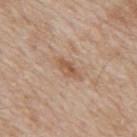<tbp_lesion>
<biopsy_status>not biopsied; imaged during a skin examination</biopsy_status>
<image>
  <source>total-body photography crop</source>
  <field_of_view_mm>15</field_of_view_mm>
</image>
<patient>
  <sex>male</sex>
  <age_approx>65</age_approx>
</patient>
<lighting>white-light</lighting>
<lesion_size>
  <long_diameter_mm_approx>3.0</long_diameter_mm_approx>
</lesion_size>
<site>mid back</site>
<automated_metrics>
  <cielab_L>55</cielab_L>
  <cielab_a>19</cielab_a>
  <cielab_b>31</cielab_b>
  <vs_skin_darker_L>9.0</vs_skin_darker_L>
  <vs_skin_contrast_norm>7.0</vs_skin_contrast_norm>
  <border_irregularity_0_10>2.0</border_irregularity_0_10>
</automated_metrics>
</tbp_lesion>